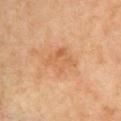Q: Was this lesion biopsied?
A: imaged on a skin check; not biopsied
Q: How large is the lesion?
A: about 4 mm
Q: What is the anatomic site?
A: the left upper arm
Q: Who is the patient?
A: female, aged around 70
Q: Illumination type?
A: cross-polarized illumination
Q: What is the imaging modality?
A: 15 mm crop, total-body photography